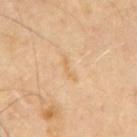Located on the mid back. The tile uses cross-polarized illumination. A 15 mm crop from a total-body photograph taken for skin-cancer surveillance. A male patient about 70 years old.